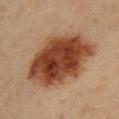subject — female, in their mid-40s | site — the left upper arm | image source — total-body-photography crop, ~15 mm field of view | image-analysis metrics — a footprint of about 43 mm², a shape eccentricity near 0.8, and a symmetry-axis asymmetry near 0.15; a mean CIELAB color near L≈37 a*≈21 b*≈29 and a lesion–skin lightness drop of about 15; a border-irregularity index near 2/10, a within-lesion color-variation index near 7/10, and a peripheral color-asymmetry measure near 2; an automated nevus-likeness rating near 100 out of 100.